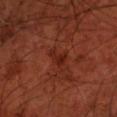<tbp_lesion>
  <biopsy_status>not biopsied; imaged during a skin examination</biopsy_status>
  <automated_metrics>
    <area_mm2_approx>3.5</area_mm2_approx>
    <eccentricity>0.85</eccentricity>
    <shape_asymmetry>0.5</shape_asymmetry>
    <cielab_L>24</cielab_L>
    <cielab_a>27</cielab_a>
    <cielab_b>28</cielab_b>
    <vs_skin_darker_L>6.0</vs_skin_darker_L>
    <vs_skin_contrast_norm>6.5</vs_skin_contrast_norm>
    <border_irregularity_0_10>5.0</border_irregularity_0_10>
    <color_variation_0_10>1.0</color_variation_0_10>
    <peripheral_color_asymmetry>0.5</peripheral_color_asymmetry>
    <nevus_likeness_0_100>0</nevus_likeness_0_100>
    <lesion_detection_confidence_0_100>100</lesion_detection_confidence_0_100>
  </automated_metrics>
  <lesion_size>
    <long_diameter_mm_approx>3.0</long_diameter_mm_approx>
  </lesion_size>
  <lighting>cross-polarized</lighting>
  <site>front of the torso</site>
  <image>
    <source>total-body photography crop</source>
    <field_of_view_mm>15</field_of_view_mm>
  </image>
  <patient>
    <sex>male</sex>
    <age_approx>70</age_approx>
  </patient>
</tbp_lesion>